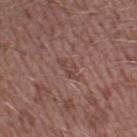The lesion was tiled from a total-body skin photograph and was not biopsied.
Approximately 3.5 mm at its widest.
The tile uses white-light illumination.
Automated tile analysis of the lesion measured an eccentricity of roughly 0.9 and two-axis asymmetry of about 0.35. And it measured border irregularity of about 4 on a 0–10 scale, a within-lesion color-variation index near 1.5/10, and radial color variation of about 0.5.
A male patient aged 43–47.
This image is a 15 mm lesion crop taken from a total-body photograph.
Located on the left thigh.